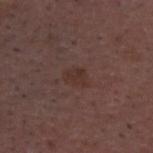Notes:
- biopsy status: total-body-photography surveillance lesion; no biopsy
- location: the head or neck
- automated lesion analysis: border irregularity of about 3 on a 0–10 scale, internal color variation of about 1.5 on a 0–10 scale, and radial color variation of about 0.5
- diameter: ~3 mm (longest diameter)
- tile lighting: white-light illumination
- imaging modality: ~15 mm crop, total-body skin-cancer survey
- subject: male, aged approximately 50